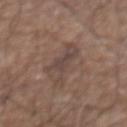| key | value |
|---|---|
| notes | total-body-photography surveillance lesion; no biopsy |
| patient | male, aged 73–77 |
| illumination | white-light illumination |
| anatomic site | the abdomen |
| lesion diameter | ~5.5 mm (longest diameter) |
| image | ~15 mm crop, total-body skin-cancer survey |
| automated metrics | an area of roughly 9 mm², a shape eccentricity near 0.85, and two-axis asymmetry of about 0.55; a lesion color around L≈44 a*≈15 b*≈21 in CIELAB, a lesion–skin lightness drop of about 7, and a normalized lesion–skin contrast near 6; a nevus-likeness score of about 0/100 and lesion-presence confidence of about 95/100 |